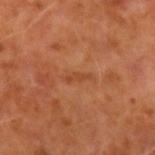biopsy_status: not biopsied; imaged during a skin examination
image:
  source: total-body photography crop
  field_of_view_mm: 15
patient:
  sex: male
  age_approx: 60
site: left forearm